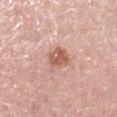Q: Is there a histopathology result?
A: total-body-photography surveillance lesion; no biopsy
Q: What is the imaging modality?
A: ~15 mm crop, total-body skin-cancer survey
Q: What is the anatomic site?
A: the right lower leg
Q: Patient demographics?
A: male, roughly 65 years of age